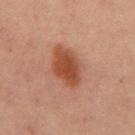• workup — catalogued during a skin exam; not biopsied
• patient — male, in their mid-60s
• lesion diameter — ~5.5 mm (longest diameter)
• acquisition — ~15 mm tile from a whole-body skin photo
• automated metrics — a border-irregularity rating of about 1.5/10, a within-lesion color-variation index near 3/10, and radial color variation of about 1; an automated nevus-likeness rating near 100 out of 100 and a detector confidence of about 100 out of 100 that the crop contains a lesion
• location — the abdomen